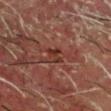workup: total-body-photography surveillance lesion; no biopsy | acquisition: 15 mm crop, total-body photography | automated lesion analysis: an area of roughly 3 mm², an outline eccentricity of about 0.8 (0 = round, 1 = elongated), and two-axis asymmetry of about 0.45; a lesion color around L≈26 a*≈21 b*≈23 in CIELAB, roughly 7 lightness units darker than nearby skin, and a normalized border contrast of about 7.5 | body site: the head or neck | patient: male, aged approximately 70 | lighting: cross-polarized | size: about 2.5 mm.